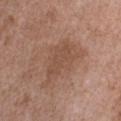Assessment: Captured during whole-body skin photography for melanoma surveillance; the lesion was not biopsied. Background: Cropped from a whole-body photographic skin survey; the tile spans about 15 mm. Automated tile analysis of the lesion measured an automated nevus-likeness rating near 0 out of 100 and lesion-presence confidence of about 100/100. The subject is a male in their 50s. Captured under white-light illumination. From the chest.